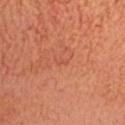Recorded during total-body skin imaging; not selected for excision or biopsy.